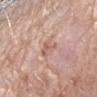Captured during whole-body skin photography for melanoma surveillance; the lesion was not biopsied. This is a white-light tile. The lesion-visualizer software estimated an average lesion color of about L≈60 a*≈20 b*≈27 (CIELAB), a lesion–skin lightness drop of about 8, and a normalized border contrast of about 5.5. And it measured a border-irregularity rating of about 3/10, internal color variation of about 2.5 on a 0–10 scale, and a peripheral color-asymmetry measure near 1. A close-up tile cropped from a whole-body skin photograph, about 15 mm across. The patient is a female in their 70s. The lesion is on the left forearm.